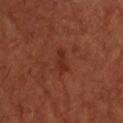Notes:
• notes: catalogued during a skin exam; not biopsied
• anatomic site: the chest
• tile lighting: cross-polarized
• imaging modality: ~15 mm tile from a whole-body skin photo
• patient: male, about 65 years old
• lesion diameter: ≈3.5 mm